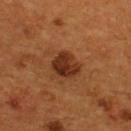Findings:
– workup · catalogued during a skin exam; not biopsied
– illumination · cross-polarized illumination
– subject · male, in their mid- to late 50s
– diameter · ≈3 mm
– location · the upper back
– acquisition · ~15 mm tile from a whole-body skin photo